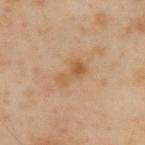subject=male, in their mid-50s | site=the upper back | lesion size=≈4 mm | image=~15 mm tile from a whole-body skin photo.